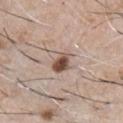This lesion was catalogued during total-body skin photography and was not selected for biopsy. The total-body-photography lesion software estimated an area of roughly 8 mm² and a symmetry-axis asymmetry near 0.3. And it measured an automated nevus-likeness rating near 100 out of 100 and a detector confidence of about 100 out of 100 that the crop contains a lesion. A male subject, aged 58–62. A region of skin cropped from a whole-body photographic capture, roughly 15 mm wide. Located on the chest. Measured at roughly 3 mm in maximum diameter.